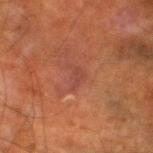The lesion was photographed on a routine skin check and not biopsied; there is no pathology result.
The lesion's longest dimension is about 3 mm.
The subject is a male aged around 65.
The lesion is on the left lower leg.
Automated image analysis of the tile measured an average lesion color of about L≈35 a*≈23 b*≈24 (CIELAB), a lesion–skin lightness drop of about 5, and a normalized lesion–skin contrast near 5.5. The software also gave a nevus-likeness score of about 0/100 and lesion-presence confidence of about 95/100.
A 15 mm close-up extracted from a 3D total-body photography capture.
The tile uses cross-polarized illumination.